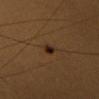Q: Is there a histopathology result?
A: imaged on a skin check; not biopsied
Q: Where on the body is the lesion?
A: the head or neck
Q: Automated lesion metrics?
A: an area of roughly 2.5 mm² and an outline eccentricity of about 0.6 (0 = round, 1 = elongated); a lesion color around L≈26 a*≈17 b*≈27 in CIELAB and a lesion–skin lightness drop of about 10; border irregularity of about 2 on a 0–10 scale, a within-lesion color-variation index near 3.5/10, and peripheral color asymmetry of about 1; an automated nevus-likeness rating near 95 out of 100 and a lesion-detection confidence of about 100/100
Q: What are the patient's age and sex?
A: female, aged around 30
Q: Illumination type?
A: cross-polarized illumination
Q: How was this image acquired?
A: ~15 mm crop, total-body skin-cancer survey